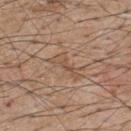biopsy_status: not biopsied; imaged during a skin examination
image:
  source: total-body photography crop
  field_of_view_mm: 15
site: upper back
patient:
  sex: male
  age_approx: 55
lesion_size:
  long_diameter_mm_approx: 4.0